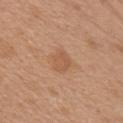Notes:
• workup · imaged on a skin check; not biopsied
• imaging modality · 15 mm crop, total-body photography
• patient · female, about 40 years old
• site · the front of the torso